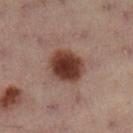Q: Was a biopsy performed?
A: catalogued during a skin exam; not biopsied
Q: How was this image acquired?
A: 15 mm crop, total-body photography
Q: Patient demographics?
A: female, aged approximately 55
Q: Lesion location?
A: the left leg
Q: Illumination type?
A: cross-polarized illumination